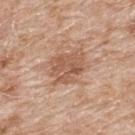Imaged during a routine full-body skin examination; the lesion was not biopsied and no histopathology is available. An algorithmic analysis of the crop reported a lesion area of about 11 mm², a shape eccentricity near 0.65, and a symmetry-axis asymmetry near 0.25. The analysis additionally found an average lesion color of about L≈56 a*≈21 b*≈31 (CIELAB), a lesion–skin lightness drop of about 10, and a lesion-to-skin contrast of about 6.5 (normalized; higher = more distinct). The software also gave internal color variation of about 3 on a 0–10 scale and a peripheral color-asymmetry measure near 1. A close-up tile cropped from a whole-body skin photograph, about 15 mm across. The tile uses white-light illumination. A male subject, aged 78–82. Located on the upper back. The lesion's longest dimension is about 4.5 mm.